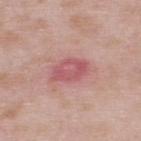Findings:
– notes — total-body-photography surveillance lesion; no biopsy
– body site — the upper back
– subject — male, approximately 55 years of age
– acquisition — ~15 mm tile from a whole-body skin photo